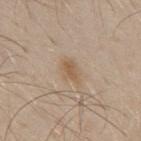{
  "biopsy_status": "not biopsied; imaged during a skin examination",
  "lesion_size": {
    "long_diameter_mm_approx": 2.5
  },
  "image": {
    "source": "total-body photography crop",
    "field_of_view_mm": 15
  },
  "lighting": "white-light",
  "site": "mid back",
  "patient": {
    "sex": "male",
    "age_approx": 30
  }
}